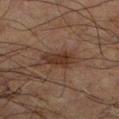Q: Was a biopsy performed?
A: total-body-photography surveillance lesion; no biopsy
Q: What is the imaging modality?
A: 15 mm crop, total-body photography
Q: What is the lesion's diameter?
A: ≈4 mm
Q: What is the anatomic site?
A: the right forearm
Q: Patient demographics?
A: male, roughly 70 years of age
Q: Illumination type?
A: cross-polarized illumination